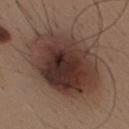Clinical impression: No biopsy was performed on this lesion — it was imaged during a full skin examination and was not determined to be concerning. Acquisition and patient details: From the mid back. Automated tile analysis of the lesion measured a footprint of about 50 mm², a shape eccentricity near 0.7, and a shape-asymmetry score of about 0.1 (0 = symmetric). It also reported a nevus-likeness score of about 55/100 and a lesion-detection confidence of about 100/100. A lesion tile, about 15 mm wide, cut from a 3D total-body photograph. This is a white-light tile. A male subject, aged 38–42. Approximately 10 mm at its widest.